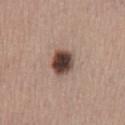The lesion was tiled from a total-body skin photograph and was not biopsied. Captured under white-light illumination. Approximately 3.5 mm at its widest. The subject is a male about 45 years old. Automated image analysis of the tile measured a lesion color around L≈42 a*≈17 b*≈22 in CIELAB, roughly 20 lightness units darker than nearby skin, and a lesion-to-skin contrast of about 14.5 (normalized; higher = more distinct). It also reported border irregularity of about 1.5 on a 0–10 scale and a within-lesion color-variation index near 6.5/10. The software also gave an automated nevus-likeness rating near 95 out of 100 and a detector confidence of about 100 out of 100 that the crop contains a lesion. The lesion is located on the back. A 15 mm close-up tile from a total-body photography series done for melanoma screening.